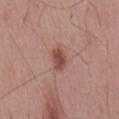Notes:
• notes: catalogued during a skin exam; not biopsied